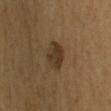• follow-up: catalogued during a skin exam; not biopsied
• lighting: cross-polarized
• patient: male, aged 63 to 67
• imaging modality: 15 mm crop, total-body photography
• anatomic site: the arm
• diameter: ~3 mm (longest diameter)